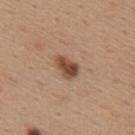Q: Was this lesion biopsied?
A: catalogued during a skin exam; not biopsied
Q: How was this image acquired?
A: 15 mm crop, total-body photography
Q: What is the anatomic site?
A: the upper back
Q: What did automated image analysis measure?
A: a lesion color around L≈48 a*≈20 b*≈29 in CIELAB, roughly 14 lightness units darker than nearby skin, and a normalized border contrast of about 10; a color-variation rating of about 4/10 and peripheral color asymmetry of about 1
Q: How was the tile lit?
A: white-light
Q: Patient demographics?
A: female, aged 38 to 42
Q: Lesion size?
A: ≈3 mm